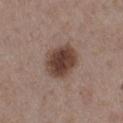  biopsy_status: not biopsied; imaged during a skin examination
  image:
    source: total-body photography crop
    field_of_view_mm: 15
  patient:
    sex: male
    age_approx: 55
  site: front of the torso